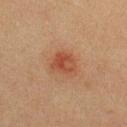follow-up — catalogued during a skin exam; not biopsied
tile lighting — cross-polarized illumination
subject — male, aged 38 to 42
location — the front of the torso
image — 15 mm crop, total-body photography
automated metrics — an outline eccentricity of about 0.55 (0 = round, 1 = elongated) and a symmetry-axis asymmetry near 0.2; a mean CIELAB color near L≈39 a*≈22 b*≈28 and a normalized border contrast of about 7; an automated nevus-likeness rating near 10 out of 100 and a lesion-detection confidence of about 100/100